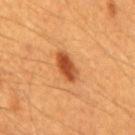Case summary:
* notes: catalogued during a skin exam; not biopsied
* image: 15 mm crop, total-body photography
* subject: male, aged approximately 60
* body site: the back